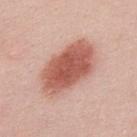The lesion was tiled from a total-body skin photograph and was not biopsied.
A male patient, roughly 25 years of age.
The lesion is located on the upper back.
A 15 mm close-up extracted from a 3D total-body photography capture.